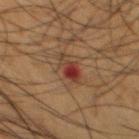This lesion was catalogued during total-body skin photography and was not selected for biopsy. Located on the front of the torso. A close-up tile cropped from a whole-body skin photograph, about 15 mm across. Measured at roughly 4 mm in maximum diameter. The lesion-visualizer software estimated a footprint of about 5.5 mm², an outline eccentricity of about 0.9 (0 = round, 1 = elongated), and a shape-asymmetry score of about 0.25 (0 = symmetric). The analysis additionally found an average lesion color of about L≈34 a*≈22 b*≈26 (CIELAB) and about 9 CIELAB-L* units darker than the surrounding skin. The software also gave a border-irregularity index near 4/10 and radial color variation of about 3. The patient is a male in their mid-50s.